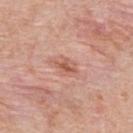Findings:
- notes — imaged on a skin check; not biopsied
- location — the back
- size — ≈3 mm
- imaging modality — ~15 mm crop, total-body skin-cancer survey
- subject — female, aged approximately 40
- automated metrics — a footprint of about 4 mm², an eccentricity of roughly 0.75, and two-axis asymmetry of about 0.25; a border-irregularity rating of about 3/10, a within-lesion color-variation index near 4.5/10, and peripheral color asymmetry of about 1.5